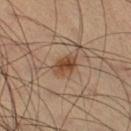This lesion was catalogued during total-body skin photography and was not selected for biopsy. A lesion tile, about 15 mm wide, cut from a 3D total-body photograph. From the right thigh. A male subject aged 63 to 67. This is a cross-polarized tile. Measured at roughly 3 mm in maximum diameter.